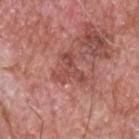Findings:
* workup — imaged on a skin check; not biopsied
* image — ~15 mm crop, total-body skin-cancer survey
* automated metrics — a lesion area of about 6.5 mm², an eccentricity of roughly 0.55, and two-axis asymmetry of about 0.8; a normalized border contrast of about 6; a detector confidence of about 100 out of 100 that the crop contains a lesion
* lesion diameter — ~4 mm (longest diameter)
* anatomic site — the upper back
* subject — male, aged 58–62